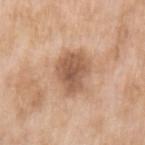{"biopsy_status": "not biopsied; imaged during a skin examination"}